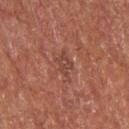Imaged during a routine full-body skin examination; the lesion was not biopsied and no histopathology is available.
On the back.
Cropped from a total-body skin-imaging series; the visible field is about 15 mm.
This is a cross-polarized tile.
A male subject in their mid-50s.
An algorithmic analysis of the crop reported an area of roughly 4.5 mm² and a shape eccentricity near 0.85. The analysis additionally found a lesion color around L≈38 a*≈21 b*≈24 in CIELAB.
The recorded lesion diameter is about 3.5 mm.